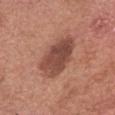follow-up = no biopsy performed (imaged during a skin exam)
patient = male, aged around 75
size = ≈5 mm
lighting = white-light
image source = ~15 mm crop, total-body skin-cancer survey
site = the head or neck